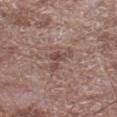notes: no biopsy performed (imaged during a skin exam)
anatomic site: the left lower leg
image-analysis metrics: an outline eccentricity of about 0.75 (0 = round, 1 = elongated) and a symmetry-axis asymmetry near 0.7; an average lesion color of about L≈47 a*≈18 b*≈22 (CIELAB), about 8 CIELAB-L* units darker than the surrounding skin, and a normalized lesion–skin contrast near 6.5; border irregularity of about 7.5 on a 0–10 scale and peripheral color asymmetry of about 0.5; an automated nevus-likeness rating near 0 out of 100 and a lesion-detection confidence of about 100/100
subject: male, approximately 70 years of age
imaging modality: ~15 mm crop, total-body skin-cancer survey